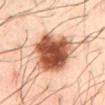– patient: male, about 50 years old
– illumination: cross-polarized illumination
– size: ≈7 mm
– location: the mid back
– image source: ~15 mm tile from a whole-body skin photo